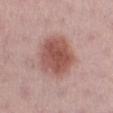Clinical impression:
The lesion was photographed on a routine skin check and not biopsied; there is no pathology result.
Image and clinical context:
The tile uses white-light illumination. The lesion's longest dimension is about 5.5 mm. Located on the right thigh. A 15 mm crop from a total-body photograph taken for skin-cancer surveillance. The patient is a female aged 28–32.